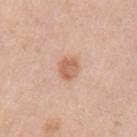Impression:
The lesion was photographed on a routine skin check and not biopsied; there is no pathology result.
Clinical summary:
The lesion-visualizer software estimated a lesion color around L≈62 a*≈22 b*≈33 in CIELAB, about 10 CIELAB-L* units darker than the surrounding skin, and a lesion-to-skin contrast of about 7 (normalized; higher = more distinct). It also reported a border-irregularity index near 2.5/10, a within-lesion color-variation index near 2.5/10, and peripheral color asymmetry of about 1. And it measured a classifier nevus-likeness of about 85/100. A region of skin cropped from a whole-body photographic capture, roughly 15 mm wide. Imaged with white-light lighting. Approximately 3 mm at its widest. A female patient aged approximately 40. On the left upper arm.